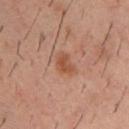Clinical summary: Approximately 3 mm at its widest. The total-body-photography lesion software estimated border irregularity of about 3 on a 0–10 scale, a color-variation rating of about 2/10, and peripheral color asymmetry of about 0.5. The software also gave a nevus-likeness score of about 75/100 and lesion-presence confidence of about 100/100. On the upper back. Imaged with cross-polarized lighting. A roughly 15 mm field-of-view crop from a total-body skin photograph. A male patient in their 40s.